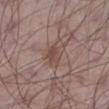Notes:
* follow-up · imaged on a skin check; not biopsied
* patient · male, about 55 years old
* image-analysis metrics · a footprint of about 6 mm² and a shape-asymmetry score of about 0.3 (0 = symmetric); a mean CIELAB color near L≈47 a*≈17 b*≈23, a lesion–skin lightness drop of about 7, and a normalized border contrast of about 6; a border-irregularity index near 3/10, a within-lesion color-variation index near 3.5/10, and radial color variation of about 1.5; an automated nevus-likeness rating near 5 out of 100
* acquisition · ~15 mm tile from a whole-body skin photo
* anatomic site · the left lower leg
* illumination · white-light illumination
* diameter · ≈3 mm